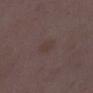Clinical impression:
This lesion was catalogued during total-body skin photography and was not selected for biopsy.
Image and clinical context:
The recorded lesion diameter is about 2.5 mm. The lesion is on the left lower leg. Imaged with white-light lighting. A female patient aged 28–32. This image is a 15 mm lesion crop taken from a total-body photograph.